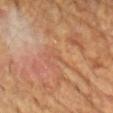Q: Was a biopsy performed?
A: imaged on a skin check; not biopsied
Q: What are the patient's age and sex?
A: male, aged 68–72
Q: Lesion location?
A: the chest
Q: How was this image acquired?
A: ~15 mm tile from a whole-body skin photo